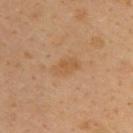Image and clinical context:
A male subject about 40 years old. The recorded lesion diameter is about 3.5 mm. A roughly 15 mm field-of-view crop from a total-body skin photograph. Imaged with cross-polarized lighting. The total-body-photography lesion software estimated a lesion color around L≈56 a*≈19 b*≈37 in CIELAB and a normalized lesion–skin contrast near 5. The analysis additionally found a classifier nevus-likeness of about 0/100 and lesion-presence confidence of about 100/100. On the back.